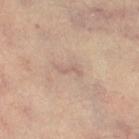Clinical impression: This lesion was catalogued during total-body skin photography and was not selected for biopsy. Background: A close-up tile cropped from a whole-body skin photograph, about 15 mm across. The patient is a female aged approximately 45. Located on the right thigh.